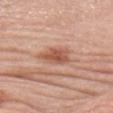Findings:
- biopsy status · imaged on a skin check; not biopsied
- site · the head or neck
- patient · female, aged 63 to 67
- image-analysis metrics · a mean CIELAB color near L≈56 a*≈24 b*≈31, a lesion–skin lightness drop of about 12, and a lesion-to-skin contrast of about 8 (normalized; higher = more distinct)
- acquisition · ~15 mm tile from a whole-body skin photo
- illumination · white-light